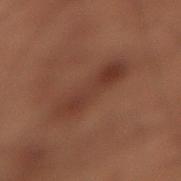Assessment:
Imaged during a routine full-body skin examination; the lesion was not biopsied and no histopathology is available.
Acquisition and patient details:
A region of skin cropped from a whole-body photographic capture, roughly 15 mm wide. Imaged with cross-polarized lighting. The lesion is on the back. The patient is a male aged 48–52. Longest diameter approximately 6 mm.